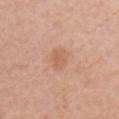  biopsy_status: not biopsied; imaged during a skin examination
  lesion_size:
    long_diameter_mm_approx: 3.0
  site: chest
  image:
    source: total-body photography crop
    field_of_view_mm: 15
  lighting: white-light
  patient:
    sex: female
    age_approx: 60